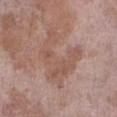follow-up — total-body-photography surveillance lesion; no biopsy
lesion diameter — ≈7.5 mm
image — total-body-photography crop, ~15 mm field of view
automated metrics — an area of roughly 17 mm², a shape eccentricity near 0.7, and a symmetry-axis asymmetry near 0.7; an automated nevus-likeness rating near 0 out of 100 and lesion-presence confidence of about 100/100
subject — female, aged 68 to 72
lighting — white-light
anatomic site — the left lower leg